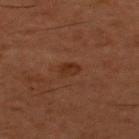Part of a total-body skin-imaging series; this lesion was reviewed on a skin check and was not flagged for biopsy.
Located on the back.
The subject is a male in their 60s.
This image is a 15 mm lesion crop taken from a total-body photograph.
Approximately 2 mm at its widest.
Imaged with cross-polarized lighting.